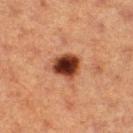Clinical impression:
Imaged during a routine full-body skin examination; the lesion was not biopsied and no histopathology is available.